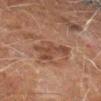No biopsy was performed on this lesion — it was imaged during a full skin examination and was not determined to be concerning. Approximately 4.5 mm at its widest. Cropped from a whole-body photographic skin survey; the tile spans about 15 mm. The subject is a male aged 58 to 62. The lesion is on the left forearm.